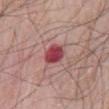notes = no biopsy performed (imaged during a skin exam)
acquisition = 15 mm crop, total-body photography
subject = male, aged approximately 60
body site = the abdomen